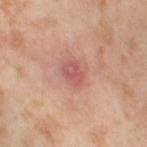This lesion was catalogued during total-body skin photography and was not selected for biopsy.